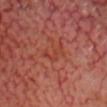  lesion_size:
    long_diameter_mm_approx: 4.0
  lighting: cross-polarized
  patient:
    age_approx: 65
  image:
    source: total-body photography crop
    field_of_view_mm: 15
  automated_metrics:
    vs_skin_darker_L: 5.0
  site: head or neck